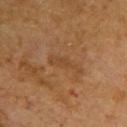follow-up: catalogued during a skin exam; not biopsied
anatomic site: the upper back
patient: female, aged approximately 55
illumination: cross-polarized illumination
lesion diameter: about 4.5 mm
image source: ~15 mm tile from a whole-body skin photo
image-analysis metrics: roughly 5 lightness units darker than nearby skin and a lesion-to-skin contrast of about 5 (normalized; higher = more distinct); peripheral color asymmetry of about 0